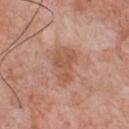Imaged during a routine full-body skin examination; the lesion was not biopsied and no histopathology is available. Measured at roughly 4.5 mm in maximum diameter. A male patient about 60 years old. A close-up tile cropped from a whole-body skin photograph, about 15 mm across. The tile uses white-light illumination. Located on the chest. Automated tile analysis of the lesion measured a lesion area of about 9.5 mm² and a shape eccentricity near 0.8. The software also gave a lesion color around L≈55 a*≈22 b*≈32 in CIELAB and a normalized border contrast of about 6.5.